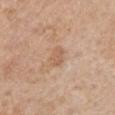Clinical impression: Part of a total-body skin-imaging series; this lesion was reviewed on a skin check and was not flagged for biopsy. Image and clinical context: The tile uses white-light illumination. A male subject roughly 75 years of age. Located on the right upper arm. The lesion's longest dimension is about 2.5 mm. A 15 mm close-up tile from a total-body photography series done for melanoma screening.